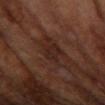workup: no biopsy performed (imaged during a skin exam) | illumination: cross-polarized illumination | patient: female, in their mid-60s | image source: 15 mm crop, total-body photography | automated metrics: a mean CIELAB color near L≈22 a*≈18 b*≈22, a lesion–skin lightness drop of about 5, and a normalized lesion–skin contrast near 6.5; internal color variation of about 2.5 on a 0–10 scale and peripheral color asymmetry of about 1 | body site: the right forearm | lesion diameter: ≈3 mm.